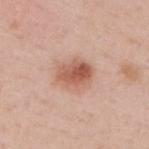Q: Lesion size?
A: ~4 mm (longest diameter)
Q: Automated lesion metrics?
A: an eccentricity of roughly 0.65 and two-axis asymmetry of about 0.15; a lesion color around L≈59 a*≈23 b*≈30 in CIELAB and a lesion–skin lightness drop of about 12
Q: What kind of image is this?
A: ~15 mm tile from a whole-body skin photo
Q: What lighting was used for the tile?
A: white-light
Q: Patient demographics?
A: male, aged around 50
Q: Lesion location?
A: the upper back